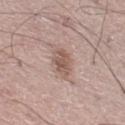  biopsy_status: not biopsied; imaged during a skin examination
  lesion_size:
    long_diameter_mm_approx: 3.5
  lighting: white-light
  image:
    source: total-body photography crop
    field_of_view_mm: 15
  patient:
    sex: male
    age_approx: 55